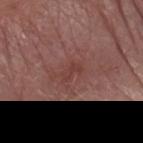{
  "biopsy_status": "not biopsied; imaged during a skin examination",
  "site": "arm",
  "lighting": "white-light",
  "patient": {
    "sex": "male",
    "age_approx": 80
  },
  "image": {
    "source": "total-body photography crop",
    "field_of_view_mm": 15
  }
}